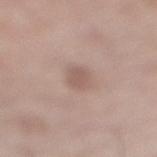Part of a total-body skin-imaging series; this lesion was reviewed on a skin check and was not flagged for biopsy.
A region of skin cropped from a whole-body photographic capture, roughly 15 mm wide.
The subject is a male aged around 70.
The tile uses white-light illumination.
Measured at roughly 3 mm in maximum diameter.
On the leg.